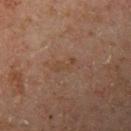Assessment:
Recorded during total-body skin imaging; not selected for excision or biopsy.
Context:
Located on the left upper arm. The subject is a male aged 43–47. An algorithmic analysis of the crop reported an average lesion color of about L≈36 a*≈15 b*≈25 (CIELAB), about 4 CIELAB-L* units darker than the surrounding skin, and a normalized lesion–skin contrast near 4.5. And it measured peripheral color asymmetry of about 0. A lesion tile, about 15 mm wide, cut from a 3D total-body photograph.